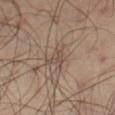  biopsy_status: not biopsied; imaged during a skin examination
  patient:
    sex: male
    age_approx: 60
  lesion_size:
    long_diameter_mm_approx: 3.5
  automated_metrics:
    cielab_L: 46
    cielab_a: 13
    cielab_b: 23
    vs_skin_darker_L: 7.0
    vs_skin_contrast_norm: 5.5
    color_variation_0_10: 2.0
    peripheral_color_asymmetry: 0.5
    nevus_likeness_0_100: 0
    lesion_detection_confidence_0_100: 60
  lighting: cross-polarized
  site: right thigh
  image:
    source: total-body photography crop
    field_of_view_mm: 15